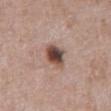biopsy_status: not biopsied; imaged during a skin examination
site: abdomen
image:
  source: total-body photography crop
  field_of_view_mm: 15
patient:
  sex: male
  age_approx: 70